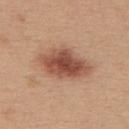{
  "biopsy_status": "not biopsied; imaged during a skin examination",
  "lighting": "white-light",
  "patient": {
    "sex": "female",
    "age_approx": 45
  },
  "lesion_size": {
    "long_diameter_mm_approx": 6.5
  },
  "image": {
    "source": "total-body photography crop",
    "field_of_view_mm": 15
  },
  "automated_metrics": {
    "area_mm2_approx": 16.0,
    "eccentricity": 0.85,
    "shape_asymmetry": 0.2,
    "nevus_likeness_0_100": 95,
    "lesion_detection_confidence_0_100": 100
  },
  "site": "upper back"
}